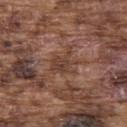The lesion was photographed on a routine skin check and not biopsied; there is no pathology result.
A 15 mm crop from a total-body photograph taken for skin-cancer surveillance.
Located on the back.
A male subject about 75 years old.